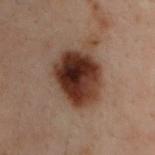The lesion was tiled from a total-body skin photograph and was not biopsied. A male subject, aged around 30. Located on the upper back. A 15 mm close-up tile from a total-body photography series done for melanoma screening.